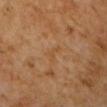Findings:
• workup · catalogued during a skin exam; not biopsied
• lesion diameter · ~2.5 mm (longest diameter)
• TBP lesion metrics · a shape eccentricity near 0.9 and a shape-asymmetry score of about 0.6 (0 = symmetric); about 4 CIELAB-L* units darker than the surrounding skin and a normalized lesion–skin contrast near 4.5
• anatomic site · the head or neck
• acquisition · ~15 mm crop, total-body skin-cancer survey
• patient · male, aged 68–72